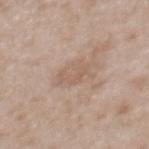Impression:
Imaged during a routine full-body skin examination; the lesion was not biopsied and no histopathology is available.
Context:
Automated tile analysis of the lesion measured a footprint of about 5 mm² and an outline eccentricity of about 0.8 (0 = round, 1 = elongated). It also reported a mean CIELAB color near L≈58 a*≈16 b*≈27, a lesion–skin lightness drop of about 6, and a lesion-to-skin contrast of about 4.5 (normalized; higher = more distinct). It also reported a border-irregularity rating of about 7.5/10, a color-variation rating of about 0/10, and radial color variation of about 0. The lesion is located on the mid back. This is a white-light tile. The subject is a female roughly 30 years of age. Cropped from a whole-body photographic skin survey; the tile spans about 15 mm.